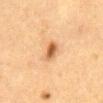Recorded during total-body skin imaging; not selected for excision or biopsy. From the abdomen. Imaged with cross-polarized lighting. This image is a 15 mm lesion crop taken from a total-body photograph. Automated image analysis of the tile measured an area of roughly 4 mm² and a shape-asymmetry score of about 0.2 (0 = symmetric). The analysis additionally found about 13 CIELAB-L* units darker than the surrounding skin. The patient is a female aged 58–62.